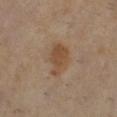follow-up — catalogued during a skin exam; not biopsied
image — total-body-photography crop, ~15 mm field of view
illumination — cross-polarized
patient — female, about 50 years old
site — the right lower leg